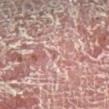Part of a total-body skin-imaging series; this lesion was reviewed on a skin check and was not flagged for biopsy.
A male patient roughly 55 years of age.
Captured under cross-polarized illumination.
The lesion is located on the right lower leg.
A lesion tile, about 15 mm wide, cut from a 3D total-body photograph.